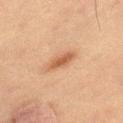Background:
Imaged with cross-polarized lighting. A male subject approximately 70 years of age. About 3.5 mm across. Cropped from a total-body skin-imaging series; the visible field is about 15 mm. The lesion is on the right thigh.